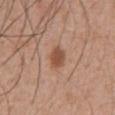A region of skin cropped from a whole-body photographic capture, roughly 15 mm wide.
On the abdomen.
The recorded lesion diameter is about 2.5 mm.
The total-body-photography lesion software estimated a color-variation rating of about 2.5/10.
The patient is a male in their mid- to late 50s.